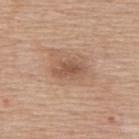Measured at roughly 4 mm in maximum diameter. The lesion-visualizer software estimated an area of roughly 7 mm², an outline eccentricity of about 0.85 (0 = round, 1 = elongated), and a symmetry-axis asymmetry near 0.2. The software also gave an average lesion color of about L≈54 a*≈19 b*≈30 (CIELAB), a lesion–skin lightness drop of about 10, and a normalized lesion–skin contrast near 7. A close-up tile cropped from a whole-body skin photograph, about 15 mm across. On the upper back. This is a white-light tile. The patient is a male aged around 60.